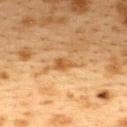Captured during whole-body skin photography for melanoma surveillance; the lesion was not biopsied.
The total-body-photography lesion software estimated a within-lesion color-variation index near 1.5/10 and peripheral color asymmetry of about 0.5. The software also gave a lesion-detection confidence of about 100/100.
The lesion is on the upper back.
Captured under cross-polarized illumination.
A 15 mm close-up tile from a total-body photography series done for melanoma screening.
A female subject in their 40s.
Approximately 2.5 mm at its widest.